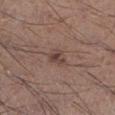Recorded during total-body skin imaging; not selected for excision or biopsy. A 15 mm close-up tile from a total-body photography series done for melanoma screening. From the left lower leg. Measured at roughly 2.5 mm in maximum diameter. The patient is a male roughly 35 years of age.